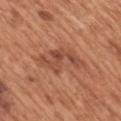Notes:
* workup — total-body-photography surveillance lesion; no biopsy
* automated metrics — a footprint of about 11 mm² and two-axis asymmetry of about 0.5; border irregularity of about 8 on a 0–10 scale, a color-variation rating of about 3.5/10, and peripheral color asymmetry of about 1; an automated nevus-likeness rating near 0 out of 100 and a detector confidence of about 100 out of 100 that the crop contains a lesion
* acquisition — 15 mm crop, total-body photography
* subject — male, approximately 65 years of age
* lesion size — ~6 mm (longest diameter)
* anatomic site — the back